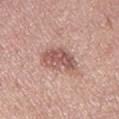follow-up = total-body-photography surveillance lesion; no biopsy | acquisition = ~15 mm tile from a whole-body skin photo | location = the leg | image-analysis metrics = a lesion color around L≈55 a*≈22 b*≈24 in CIELAB and roughly 12 lightness units darker than nearby skin; a nevus-likeness score of about 45/100 and lesion-presence confidence of about 100/100 | diameter = ≈4 mm | subject = female, aged approximately 40.